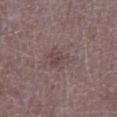No biopsy was performed on this lesion — it was imaged during a full skin examination and was not determined to be concerning.
A lesion tile, about 15 mm wide, cut from a 3D total-body photograph.
A male patient in their 50s.
The lesion is located on the left lower leg.